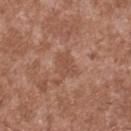Clinical impression: The lesion was tiled from a total-body skin photograph and was not biopsied. Image and clinical context: Located on the upper back. A male subject aged around 45. Longest diameter approximately 2.5 mm. A lesion tile, about 15 mm wide, cut from a 3D total-body photograph. The total-body-photography lesion software estimated a footprint of about 4 mm², an outline eccentricity of about 0.75 (0 = round, 1 = elongated), and a symmetry-axis asymmetry near 0.5. And it measured a color-variation rating of about 1/10 and a peripheral color-asymmetry measure near 0.5.